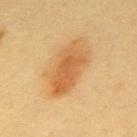follow-up — no biopsy performed (imaged during a skin exam)
tile lighting — cross-polarized
anatomic site — the mid back
patient — female, approximately 55 years of age
image — total-body-photography crop, ~15 mm field of view
automated lesion analysis — an area of roughly 17 mm² and an eccentricity of roughly 0.85; a within-lesion color-variation index near 4/10 and radial color variation of about 1.5; a nevus-likeness score of about 100/100 and a lesion-detection confidence of about 100/100